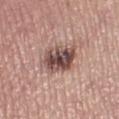Clinical impression: The lesion was tiled from a total-body skin photograph and was not biopsied. Acquisition and patient details: Automated image analysis of the tile measured internal color variation of about 8.5 on a 0–10 scale and radial color variation of about 3. A 15 mm close-up extracted from a 3D total-body photography capture. About 4 mm across. The lesion is on the left lower leg. This is a white-light tile. A female patient in their mid- to late 60s.